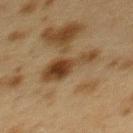Clinical impression:
This lesion was catalogued during total-body skin photography and was not selected for biopsy.
Clinical summary:
A lesion tile, about 15 mm wide, cut from a 3D total-body photograph. A female subject approximately 40 years of age. Automated image analysis of the tile measured a lesion area of about 11 mm² and an outline eccentricity of about 0.95 (0 = round, 1 = elongated). And it measured about 11 CIELAB-L* units darker than the surrounding skin and a lesion-to-skin contrast of about 10 (normalized; higher = more distinct). And it measured a classifier nevus-likeness of about 0/100 and lesion-presence confidence of about 100/100. On the upper back.